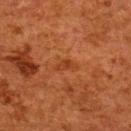biopsy status: total-body-photography surveillance lesion; no biopsy
automated metrics: a footprint of about 2.5 mm², an outline eccentricity of about 0.9 (0 = round, 1 = elongated), and a shape-asymmetry score of about 0.4 (0 = symmetric); a border-irregularity index near 4/10, internal color variation of about 0 on a 0–10 scale, and peripheral color asymmetry of about 0; an automated nevus-likeness rating near 0 out of 100 and a detector confidence of about 100 out of 100 that the crop contains a lesion
location: the upper back
lesion diameter: ≈2.5 mm
illumination: cross-polarized
acquisition: ~15 mm crop, total-body skin-cancer survey
subject: female, approximately 50 years of age